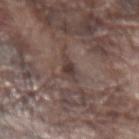Part of a total-body skin-imaging series; this lesion was reviewed on a skin check and was not flagged for biopsy. A 15 mm crop from a total-body photograph taken for skin-cancer surveillance. From the left forearm. Approximately 3 mm at its widest. This is a white-light tile. A male subject, roughly 75 years of age. The total-body-photography lesion software estimated an average lesion color of about L≈39 a*≈14 b*≈19 (CIELAB), about 8 CIELAB-L* units darker than the surrounding skin, and a normalized lesion–skin contrast near 7.5. The software also gave a border-irregularity rating of about 4.5/10, a within-lesion color-variation index near 2.5/10, and peripheral color asymmetry of about 1.